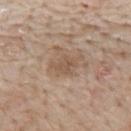Q: Is there a histopathology result?
A: total-body-photography surveillance lesion; no biopsy
Q: What is the anatomic site?
A: the mid back
Q: Patient demographics?
A: male, aged approximately 65
Q: How was this image acquired?
A: ~15 mm tile from a whole-body skin photo